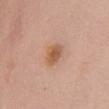Imaged during a routine full-body skin examination; the lesion was not biopsied and no histopathology is available.
The subject is a female aged 48 to 52.
From the chest.
The tile uses white-light illumination.
Automated tile analysis of the lesion measured a mean CIELAB color near L≈57 a*≈21 b*≈34, roughly 10 lightness units darker than nearby skin, and a normalized border contrast of about 7.5. The software also gave a classifier nevus-likeness of about 90/100 and a lesion-detection confidence of about 100/100.
Longest diameter approximately 3 mm.
A 15 mm close-up tile from a total-body photography series done for melanoma screening.